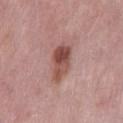biopsy status: imaged on a skin check; not biopsied | patient: male, roughly 55 years of age | site: the mid back | automated lesion analysis: an average lesion color of about L≈50 a*≈23 b*≈24 (CIELAB), roughly 13 lightness units darker than nearby skin, and a normalized lesion–skin contrast near 9 | image: ~15 mm tile from a whole-body skin photo | size: about 5 mm | lighting: white-light illumination.